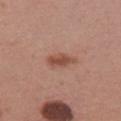| feature | finding |
|---|---|
| follow-up | catalogued during a skin exam; not biopsied |
| acquisition | 15 mm crop, total-body photography |
| subject | female, aged 23 to 27 |
| body site | the chest |
| lighting | white-light illumination |
| lesion size | ~3.5 mm (longest diameter) |
| automated lesion analysis | an eccentricity of roughly 0.9 |